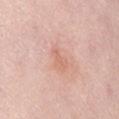biopsy_status: not biopsied; imaged during a skin examination
image:
  source: total-body photography crop
  field_of_view_mm: 15
lighting: white-light
site: abdomen
automated_metrics:
  area_mm2_approx: 3.0
  eccentricity: 0.9
  shape_asymmetry: 0.4
patient:
  sex: female
  age_approx: 60